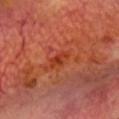Imaged during a routine full-body skin examination; the lesion was not biopsied and no histopathology is available.
A region of skin cropped from a whole-body photographic capture, roughly 15 mm wide.
Captured under cross-polarized illumination.
An algorithmic analysis of the crop reported an average lesion color of about L≈40 a*≈35 b*≈39 (CIELAB), a lesion–skin lightness drop of about 7, and a normalized lesion–skin contrast near 7. And it measured a border-irregularity rating of about 3.5/10, a within-lesion color-variation index near 2.5/10, and a peripheral color-asymmetry measure near 1. The analysis additionally found an automated nevus-likeness rating near 0 out of 100 and a detector confidence of about 100 out of 100 that the crop contains a lesion.
Measured at roughly 3 mm in maximum diameter.
The lesion is on the head or neck.
A male patient, in their mid- to late 60s.